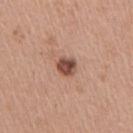notes: no biopsy performed (imaged during a skin exam)
imaging modality: ~15 mm tile from a whole-body skin photo
subject: female, roughly 45 years of age
anatomic site: the arm
tile lighting: white-light illumination
TBP lesion metrics: a footprint of about 4.5 mm², a shape eccentricity near 0.45, and a symmetry-axis asymmetry near 0.25; a within-lesion color-variation index near 4.5/10 and a peripheral color-asymmetry measure near 1.5
diameter: about 2.5 mm